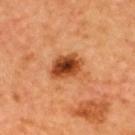Case summary:
* TBP lesion metrics · a lesion-to-skin contrast of about 11 (normalized; higher = more distinct); internal color variation of about 9 on a 0–10 scale
* subject · male, aged 63–67
* lesion diameter · ~4 mm (longest diameter)
* image · total-body-photography crop, ~15 mm field of view
* anatomic site · the upper back
* tile lighting · cross-polarized illumination